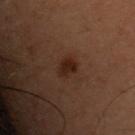This is a cross-polarized tile. The recorded lesion diameter is about 3 mm. From the chest. A male subject, aged around 30. A close-up tile cropped from a whole-body skin photograph, about 15 mm across.